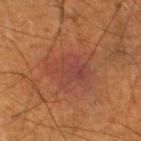The lesion was photographed on a routine skin check and not biopsied; there is no pathology result.
A male patient aged 63–67.
From the left lower leg.
The tile uses cross-polarized illumination.
Approximately 5.5 mm at its widest.
A roughly 15 mm field-of-view crop from a total-body skin photograph.